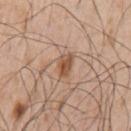workup: imaged on a skin check; not biopsied
image: ~15 mm crop, total-body skin-cancer survey
anatomic site: the left upper arm
patient: male, aged 63–67